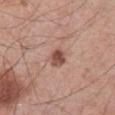Assessment: Captured during whole-body skin photography for melanoma surveillance; the lesion was not biopsied. Acquisition and patient details: The lesion is located on the abdomen. The subject is a male about 70 years old. A lesion tile, about 15 mm wide, cut from a 3D total-body photograph. The lesion's longest dimension is about 2.5 mm. This is a white-light tile.